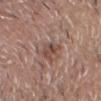{"biopsy_status": "not biopsied; imaged during a skin examination"}